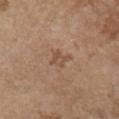The lesion was tiled from a total-body skin photograph and was not biopsied. The lesion's longest dimension is about 3 mm. This is a white-light tile. A 15 mm close-up tile from a total-body photography series done for melanoma screening. Located on the chest. A female patient approximately 65 years of age.